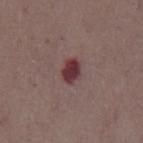Impression:
The lesion was tiled from a total-body skin photograph and was not biopsied.
Background:
This image is a 15 mm lesion crop taken from a total-body photograph. The recorded lesion diameter is about 2.5 mm. The patient is a male aged approximately 70. Captured under white-light illumination. The total-body-photography lesion software estimated a shape eccentricity near 0.7 and a shape-asymmetry score of about 0.2 (0 = symmetric). The software also gave a color-variation rating of about 3.5/10 and radial color variation of about 1. And it measured a classifier nevus-likeness of about 0/100 and lesion-presence confidence of about 100/100. On the front of the torso.